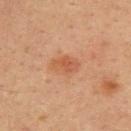Notes:
• follow-up: imaged on a skin check; not biopsied
• tile lighting: cross-polarized
• location: the back
• patient: male, in their mid-30s
• diameter: about 3.5 mm
• imaging modality: ~15 mm tile from a whole-body skin photo
• automated lesion analysis: an area of roughly 6 mm² and a shape-asymmetry score of about 0.2 (0 = symmetric); a mean CIELAB color near L≈51 a*≈24 b*≈34, a lesion–skin lightness drop of about 7, and a normalized border contrast of about 6; a classifier nevus-likeness of about 50/100 and a lesion-detection confidence of about 100/100